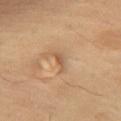follow-up: no biopsy performed (imaged during a skin exam)
size: ≈3 mm
patient: female, in their mid- to late 50s
location: the front of the torso
acquisition: ~15 mm crop, total-body skin-cancer survey
automated lesion analysis: a lesion area of about 2.5 mm², an eccentricity of roughly 0.95, and a shape-asymmetry score of about 0.4 (0 = symmetric); a mean CIELAB color near L≈57 a*≈21 b*≈34, a lesion–skin lightness drop of about 9, and a normalized border contrast of about 6; a border-irregularity index near 4.5/10 and a within-lesion color-variation index near 0/10